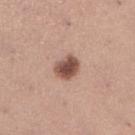Clinical impression: Recorded during total-body skin imaging; not selected for excision or biopsy. Background: A 15 mm close-up extracted from a 3D total-body photography capture. The subject is a female aged around 30. Located on the leg. The recorded lesion diameter is about 3 mm.